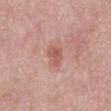Approximately 3.5 mm at its widest.
The total-body-photography lesion software estimated a border-irregularity rating of about 3.5/10 and peripheral color asymmetry of about 0.5. The analysis additionally found an automated nevus-likeness rating near 0 out of 100 and lesion-presence confidence of about 100/100.
The patient is a male aged around 55.
This is a white-light tile.
From the mid back.
A region of skin cropped from a whole-body photographic capture, roughly 15 mm wide.